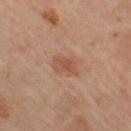Clinical impression:
The lesion was photographed on a routine skin check and not biopsied; there is no pathology result.
Clinical summary:
Approximately 3.5 mm at its widest. The lesion is on the right thigh. Imaged with cross-polarized lighting. This image is a 15 mm lesion crop taken from a total-body photograph. A female subject, roughly 50 years of age.